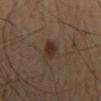– image source · 15 mm crop, total-body photography
– tile lighting · cross-polarized illumination
– diameter · ~3.5 mm (longest diameter)
– anatomic site · the mid back
– subject · male, in their 60s